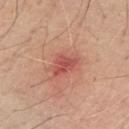{
  "biopsy_status": "not biopsied; imaged during a skin examination",
  "patient": {
    "sex": "male",
    "age_approx": 65
  },
  "image": {
    "source": "total-body photography crop",
    "field_of_view_mm": 15
  },
  "site": "arm"
}